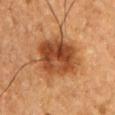notes: catalogued during a skin exam; not biopsied
body site: the arm
subject: female, roughly 55 years of age
automated lesion analysis: a symmetry-axis asymmetry near 0.15; internal color variation of about 7.5 on a 0–10 scale and radial color variation of about 3
image source: ~15 mm tile from a whole-body skin photo